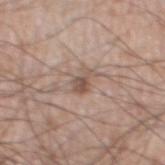Q: Was a biopsy performed?
A: total-body-photography surveillance lesion; no biopsy
Q: Patient demographics?
A: male, aged approximately 60
Q: What did automated image analysis measure?
A: border irregularity of about 4.5 on a 0–10 scale, internal color variation of about 3 on a 0–10 scale, and peripheral color asymmetry of about 1
Q: What kind of image is this?
A: total-body-photography crop, ~15 mm field of view
Q: Where on the body is the lesion?
A: the left thigh